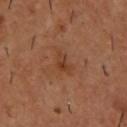Q: Was a biopsy performed?
A: no biopsy performed (imaged during a skin exam)
Q: Lesion size?
A: ≈3 mm
Q: What kind of image is this?
A: total-body-photography crop, ~15 mm field of view
Q: Illumination type?
A: cross-polarized illumination
Q: Where on the body is the lesion?
A: the upper back
Q: What are the patient's age and sex?
A: male, approximately 55 years of age
Q: Automated lesion metrics?
A: a mean CIELAB color near L≈38 a*≈22 b*≈31 and a lesion-to-skin contrast of about 6 (normalized; higher = more distinct)